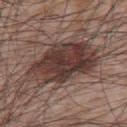| key | value |
|---|---|
| biopsy status | catalogued during a skin exam; not biopsied |
| acquisition | total-body-photography crop, ~15 mm field of view |
| illumination | white-light |
| size | ≈9.5 mm |
| body site | the upper back |
| subject | male, aged approximately 65 |